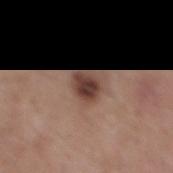The lesion was tiled from a total-body skin photograph and was not biopsied.
A close-up tile cropped from a whole-body skin photograph, about 15 mm across.
Approximately 3 mm at its widest.
A male patient in their mid- to late 50s.
On the mid back.
The tile uses white-light illumination.